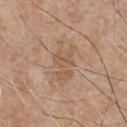Background: Captured under white-light illumination. From the chest. A male patient, roughly 65 years of age. A close-up tile cropped from a whole-body skin photograph, about 15 mm across.